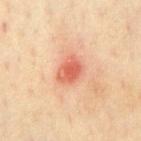Impression:
Imaged during a routine full-body skin examination; the lesion was not biopsied and no histopathology is available.
Background:
A male patient aged approximately 65. Longest diameter approximately 3.5 mm. Located on the chest. Cropped from a whole-body photographic skin survey; the tile spans about 15 mm. An algorithmic analysis of the crop reported a border-irregularity index near 2/10, internal color variation of about 4.5 on a 0–10 scale, and radial color variation of about 1. The software also gave a nevus-likeness score of about 0/100 and a detector confidence of about 100 out of 100 that the crop contains a lesion.